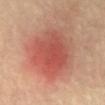patient: male, about 60 years old; acquisition: total-body-photography crop, ~15 mm field of view; location: the abdomen; illumination: cross-polarized illumination; lesion diameter: about 7.5 mm.